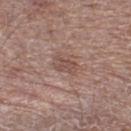The lesion was tiled from a total-body skin photograph and was not biopsied.
Automated image analysis of the tile measured a lesion area of about 4 mm² and two-axis asymmetry of about 0.3. And it measured border irregularity of about 3.5 on a 0–10 scale and radial color variation of about 0.5. The software also gave an automated nevus-likeness rating near 0 out of 100 and lesion-presence confidence of about 100/100.
Imaged with white-light lighting.
A roughly 15 mm field-of-view crop from a total-body skin photograph.
The lesion is on the leg.
The recorded lesion diameter is about 3 mm.
The subject is a male approximately 70 years of age.